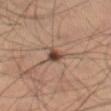The lesion is located on the left thigh. Imaged with cross-polarized lighting. A male subject roughly 65 years of age. The recorded lesion diameter is about 2.5 mm. Cropped from a total-body skin-imaging series; the visible field is about 15 mm.